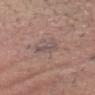Clinical impression: This lesion was catalogued during total-body skin photography and was not selected for biopsy. Background: The lesion is located on the head or neck. An algorithmic analysis of the crop reported border irregularity of about 4.5 on a 0–10 scale, a color-variation rating of about 1.5/10, and peripheral color asymmetry of about 0.5. A male subject, aged 53 to 57. This image is a 15 mm lesion crop taken from a total-body photograph. Approximately 3 mm at its widest. Imaged with white-light lighting.